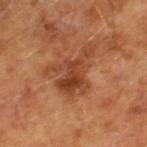Case summary:
– follow-up — no biopsy performed (imaged during a skin exam)
– lighting — cross-polarized
– imaging modality — ~15 mm tile from a whole-body skin photo
– subject — male, aged 63–67
– size — ~6 mm (longest diameter)